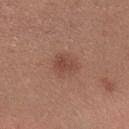No biopsy was performed on this lesion — it was imaged during a full skin examination and was not determined to be concerning.